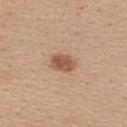workup = imaged on a skin check; not biopsied | anatomic site = the back | acquisition = total-body-photography crop, ~15 mm field of view | subject = female, aged approximately 35.